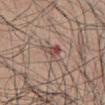workup: imaged on a skin check; not biopsied
location: the abdomen
image-analysis metrics: a lesion color around L≈50 a*≈19 b*≈23 in CIELAB, a lesion–skin lightness drop of about 10, and a lesion-to-skin contrast of about 7 (normalized; higher = more distinct); a border-irregularity index near 5/10, internal color variation of about 9 on a 0–10 scale, and radial color variation of about 4.5
subject: male, aged approximately 35
illumination: white-light illumination
lesion diameter: ~3 mm (longest diameter)
image source: ~15 mm crop, total-body skin-cancer survey